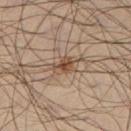Imaged during a routine full-body skin examination; the lesion was not biopsied and no histopathology is available.
The patient is a male approximately 45 years of age.
A roughly 15 mm field-of-view crop from a total-body skin photograph.
The lesion's longest dimension is about 3 mm.
From the left lower leg.